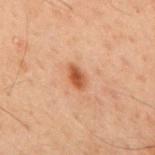Assessment:
No biopsy was performed on this lesion — it was imaged during a full skin examination and was not determined to be concerning.
Context:
Longest diameter approximately 2.5 mm. A male patient, aged 58–62. The lesion is located on the upper back. A lesion tile, about 15 mm wide, cut from a 3D total-body photograph. Automated image analysis of the tile measured a footprint of about 3.5 mm², a shape eccentricity near 0.8, and a shape-asymmetry score of about 0.3 (0 = symmetric). It also reported an average lesion color of about L≈42 a*≈21 b*≈31 (CIELAB), roughly 11 lightness units darker than nearby skin, and a lesion-to-skin contrast of about 9 (normalized; higher = more distinct). The tile uses cross-polarized illumination.